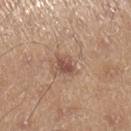{
  "biopsy_status": "not biopsied; imaged during a skin examination",
  "image": {
    "source": "total-body photography crop",
    "field_of_view_mm": 15
  },
  "lighting": "white-light",
  "lesion_size": {
    "long_diameter_mm_approx": 2.5
  },
  "patient": {
    "sex": "male",
    "age_approx": 40
  },
  "site": "right lower leg"
}